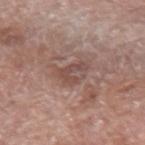Part of a total-body skin-imaging series; this lesion was reviewed on a skin check and was not flagged for biopsy. Measured at roughly 3.5 mm in maximum diameter. A male patient aged 58–62. The tile uses white-light illumination. An algorithmic analysis of the crop reported a border-irregularity rating of about 4.5/10, a color-variation rating of about 4.5/10, and a peripheral color-asymmetry measure near 1.5. The analysis additionally found an automated nevus-likeness rating near 0 out of 100 and a detector confidence of about 100 out of 100 that the crop contains a lesion. A 15 mm close-up tile from a total-body photography series done for melanoma screening. Located on the right upper arm.